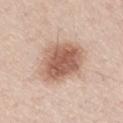This image is a 15 mm lesion crop taken from a total-body photograph.
The lesion is located on the right thigh.
The recorded lesion diameter is about 5 mm.
Imaged with white-light lighting.
A male patient in their 60s.